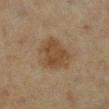Findings:
– biopsy status: imaged on a skin check; not biopsied
– imaging modality: total-body-photography crop, ~15 mm field of view
– site: the left lower leg
– patient: female, aged around 55